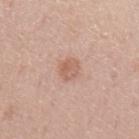Q: Was this lesion biopsied?
A: total-body-photography surveillance lesion; no biopsy
Q: What is the imaging modality?
A: total-body-photography crop, ~15 mm field of view
Q: What are the patient's age and sex?
A: male, approximately 45 years of age
Q: What did automated image analysis measure?
A: a shape-asymmetry score of about 0.2 (0 = symmetric); a border-irregularity rating of about 2/10, internal color variation of about 2.5 on a 0–10 scale, and peripheral color asymmetry of about 1
Q: Lesion location?
A: the left upper arm